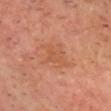biopsy status=catalogued during a skin exam; not biopsied
image source=~15 mm crop, total-body skin-cancer survey
lesion diameter=about 4 mm
tile lighting=cross-polarized
subject=male, about 70 years old
automated metrics=about 6 CIELAB-L* units darker than the surrounding skin and a normalized lesion–skin contrast near 5; a border-irregularity rating of about 5.5/10, a within-lesion color-variation index near 1.5/10, and peripheral color asymmetry of about 0.5
anatomic site=the head or neck